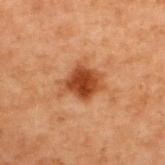Case summary:
- follow-up · catalogued during a skin exam; not biopsied
- diameter · ~4.5 mm (longest diameter)
- tile lighting · cross-polarized
- acquisition · 15 mm crop, total-body photography
- automated lesion analysis · a mean CIELAB color near L≈36 a*≈22 b*≈31 and a normalized border contrast of about 9.5; a border-irregularity index near 3/10, a within-lesion color-variation index near 3.5/10, and a peripheral color-asymmetry measure near 1
- anatomic site · the upper back
- subject · male, aged approximately 70